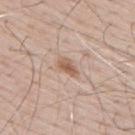Clinical impression:
Captured during whole-body skin photography for melanoma surveillance; the lesion was not biopsied.
Clinical summary:
The lesion is located on the back. Measured at roughly 3 mm in maximum diameter. The patient is a male aged 68 to 72. A region of skin cropped from a whole-body photographic capture, roughly 15 mm wide. This is a white-light tile.